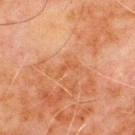Impression:
Part of a total-body skin-imaging series; this lesion was reviewed on a skin check and was not flagged for biopsy.
Image and clinical context:
About 2.5 mm across. A region of skin cropped from a whole-body photographic capture, roughly 15 mm wide. Automated tile analysis of the lesion measured a mean CIELAB color near L≈47 a*≈24 b*≈35. The software also gave border irregularity of about 5.5 on a 0–10 scale, a color-variation rating of about 0/10, and peripheral color asymmetry of about 0. The subject is a male aged 68–72. On the chest.